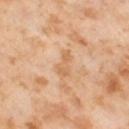| feature | finding |
|---|---|
| notes | catalogued during a skin exam; not biopsied |
| size | ≈3.5 mm |
| subject | female, aged 53–57 |
| image source | 15 mm crop, total-body photography |
| TBP lesion metrics | a lesion–skin lightness drop of about 8 and a normalized lesion–skin contrast near 5.5; a border-irregularity index near 5/10, a color-variation rating of about 1/10, and peripheral color asymmetry of about 0 |
| illumination | cross-polarized illumination |
| site | the left thigh |